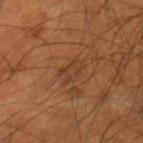This lesion was catalogued during total-body skin photography and was not selected for biopsy.
The lesion is located on the right lower leg.
Imaged with cross-polarized lighting.
Measured at roughly 3.5 mm in maximum diameter.
The total-body-photography lesion software estimated an area of roughly 5.5 mm², a shape eccentricity near 0.75, and a symmetry-axis asymmetry near 0.25. It also reported a lesion color around L≈38 a*≈20 b*≈32 in CIELAB and roughly 6 lightness units darker than nearby skin. The analysis additionally found a nevus-likeness score of about 0/100 and a lesion-detection confidence of about 100/100.
A male patient, aged around 55.
A lesion tile, about 15 mm wide, cut from a 3D total-body photograph.